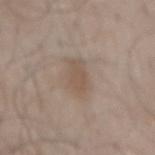Clinical impression: No biopsy was performed on this lesion — it was imaged during a full skin examination and was not determined to be concerning. Background: The lesion's longest dimension is about 4.5 mm. The lesion-visualizer software estimated an area of roughly 8.5 mm² and a shape eccentricity near 0.85. And it measured a border-irregularity rating of about 2.5/10, internal color variation of about 2 on a 0–10 scale, and peripheral color asymmetry of about 0.5. From the mid back. A 15 mm close-up tile from a total-body photography series done for melanoma screening. A male subject, aged 53–57.